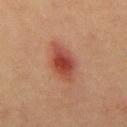Notes:
- follow-up · catalogued during a skin exam; not biopsied
- anatomic site · the mid back
- patient · male, aged around 40
- lesion size · ~5.5 mm (longest diameter)
- image · ~15 mm crop, total-body skin-cancer survey